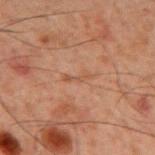Impression:
Captured during whole-body skin photography for melanoma surveillance; the lesion was not biopsied.
Background:
From the mid back. The patient is a male approximately 60 years of age. A 15 mm crop from a total-body photograph taken for skin-cancer surveillance.